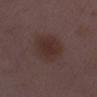notes = catalogued during a skin exam; not biopsied | subject = female, in their 30s | image = ~15 mm tile from a whole-body skin photo | size = about 4 mm | site = the right lower leg.